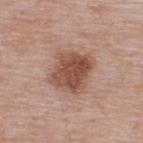{
  "biopsy_status": "not biopsied; imaged during a skin examination",
  "image": {
    "source": "total-body photography crop",
    "field_of_view_mm": 15
  },
  "automated_metrics": {
    "area_mm2_approx": 16.0,
    "eccentricity": 0.45,
    "shape_asymmetry": 0.15
  },
  "site": "upper back",
  "patient": {
    "sex": "male",
    "age_approx": 65
  },
  "lighting": "white-light"
}